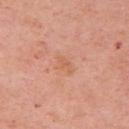The lesion was photographed on a routine skin check and not biopsied; there is no pathology result.
On the right upper arm.
The subject is a female aged approximately 65.
About 2.5 mm across.
The total-body-photography lesion software estimated a mean CIELAB color near L≈62 a*≈25 b*≈34, roughly 5 lightness units darker than nearby skin, and a normalized lesion–skin contrast near 4.5. And it measured a classifier nevus-likeness of about 0/100.
Cropped from a total-body skin-imaging series; the visible field is about 15 mm.